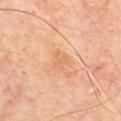| field | value |
|---|---|
| follow-up | no biopsy performed (imaged during a skin exam) |
| subject | male, about 60 years old |
| lesion size | about 2.5 mm |
| imaging modality | ~15 mm tile from a whole-body skin photo |
| site | the chest |